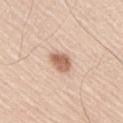| field | value |
|---|---|
| follow-up | no biopsy performed (imaged during a skin exam) |
| subject | male, aged 43–47 |
| image-analysis metrics | a footprint of about 6 mm², an eccentricity of roughly 0.55, and a shape-asymmetry score of about 0.2 (0 = symmetric); a border-irregularity index near 1.5/10, internal color variation of about 3.5 on a 0–10 scale, and a peripheral color-asymmetry measure near 1; a classifier nevus-likeness of about 95/100 and a detector confidence of about 100 out of 100 that the crop contains a lesion |
| image source | 15 mm crop, total-body photography |
| location | the right upper arm |
| lesion diameter | about 3 mm |
| illumination | white-light |